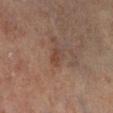Automated tile analysis of the lesion measured a lesion area of about 3.5 mm², a shape eccentricity near 0.8, and two-axis asymmetry of about 0.25. And it measured about 5 CIELAB-L* units darker than the surrounding skin and a lesion-to-skin contrast of about 5.5 (normalized; higher = more distinct). And it measured an automated nevus-likeness rating near 5 out of 100. The recorded lesion diameter is about 2.5 mm. A male patient, aged 68 to 72. From the left lower leg. Captured under cross-polarized illumination. A 15 mm close-up tile from a total-body photography series done for melanoma screening.